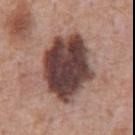Recorded during total-body skin imaging; not selected for excision or biopsy. A male patient, approximately 60 years of age. A roughly 15 mm field-of-view crop from a total-body skin photograph. Approximately 7.5 mm at its widest. The lesion is on the chest.